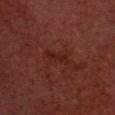Q: Is there a histopathology result?
A: catalogued during a skin exam; not biopsied
Q: Lesion size?
A: ≈3 mm
Q: Where on the body is the lesion?
A: the head or neck
Q: How was this image acquired?
A: 15 mm crop, total-body photography
Q: What are the patient's age and sex?
A: male, in their 60s